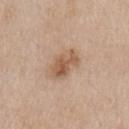Clinical impression:
This lesion was catalogued during total-body skin photography and was not selected for biopsy.
Clinical summary:
This image is a 15 mm lesion crop taken from a total-body photograph. A male patient, approximately 75 years of age. The lesion is located on the right upper arm.